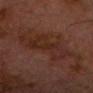Assessment: This lesion was catalogued during total-body skin photography and was not selected for biopsy. Image and clinical context: A close-up tile cropped from a whole-body skin photograph, about 15 mm across. Longest diameter approximately 7 mm. The tile uses cross-polarized illumination. On the chest. The lesion-visualizer software estimated a nevus-likeness score of about 0/100. A male subject, aged approximately 75.